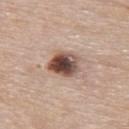follow-up — no biopsy performed (imaged during a skin exam) | subject — male, aged approximately 85 or older | site — the upper back | image — ~15 mm tile from a whole-body skin photo.